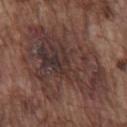{
  "biopsy_status": "not biopsied; imaged during a skin examination",
  "lighting": "white-light",
  "image": {
    "source": "total-body photography crop",
    "field_of_view_mm": 15
  },
  "site": "chest",
  "lesion_size": {
    "long_diameter_mm_approx": 11.0
  },
  "patient": {
    "sex": "male",
    "age_approx": 75
  }
}